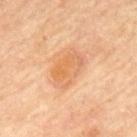| key | value |
|---|---|
| notes | imaged on a skin check; not biopsied |
| acquisition | 15 mm crop, total-body photography |
| patient | male, about 70 years old |
| lighting | cross-polarized |
| size | ≈4 mm |
| site | the mid back |